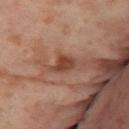{
  "biopsy_status": "not biopsied; imaged during a skin examination",
  "patient": {
    "sex": "female",
    "age_approx": 55
  },
  "site": "right thigh",
  "automated_metrics": {
    "border_irregularity_0_10": 3.5,
    "color_variation_0_10": 2.5,
    "peripheral_color_asymmetry": 0.5
  },
  "image": {
    "source": "total-body photography crop",
    "field_of_view_mm": 15
  }
}